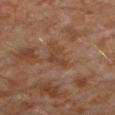biopsy status=imaged on a skin check; not biopsied | anatomic site=the leg | patient=male, aged around 30 | image=total-body-photography crop, ~15 mm field of view | lighting=cross-polarized illumination | lesion size=~3.5 mm (longest diameter) | image-analysis metrics=an automated nevus-likeness rating near 0 out of 100 and a detector confidence of about 100 out of 100 that the crop contains a lesion.